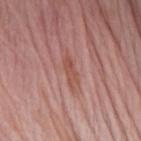Findings:
• acquisition — total-body-photography crop, ~15 mm field of view
• patient — female, aged approximately 65
• location — the arm
• illumination — white-light illumination